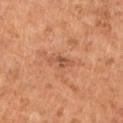biopsy status — catalogued during a skin exam; not biopsied | image source — ~15 mm crop, total-body skin-cancer survey | body site — the left lower leg | size — ~3 mm (longest diameter) | illumination — cross-polarized illumination | subject — female, aged 63–67.